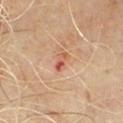Q: Was this lesion biopsied?
A: catalogued during a skin exam; not biopsied
Q: Where on the body is the lesion?
A: the mid back
Q: What is the imaging modality?
A: ~15 mm crop, total-body skin-cancer survey
Q: What are the patient's age and sex?
A: male, approximately 60 years of age
Q: How was the tile lit?
A: cross-polarized
Q: Automated lesion metrics?
A: an automated nevus-likeness rating near 0 out of 100 and lesion-presence confidence of about 100/100
Q: Lesion size?
A: ≈2.5 mm